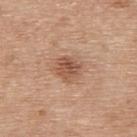The lesion was photographed on a routine skin check and not biopsied; there is no pathology result.